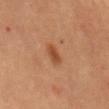image = ~15 mm crop, total-body skin-cancer survey; location = the mid back; patient = female, in their 60s; lesion size = ~3 mm (longest diameter).